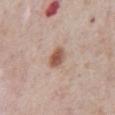No biopsy was performed on this lesion — it was imaged during a full skin examination and was not determined to be concerning.
A male subject, aged around 75.
About 3 mm across.
The tile uses white-light illumination.
From the abdomen.
Automated image analysis of the tile measured a footprint of about 5 mm² and an outline eccentricity of about 0.7 (0 = round, 1 = elongated). The analysis additionally found a nevus-likeness score of about 95/100 and a lesion-detection confidence of about 100/100.
A roughly 15 mm field-of-view crop from a total-body skin photograph.